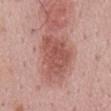<case>
<lighting>white-light</lighting>
<site>mid back</site>
<automated_metrics>
  <cielab_L>53</cielab_L>
  <cielab_a>25</cielab_a>
  <cielab_b>26</cielab_b>
  <vs_skin_darker_L>12.0</vs_skin_darker_L>
  <vs_skin_contrast_norm>8.0</vs_skin_contrast_norm>
  <nevus_likeness_0_100>75</nevus_likeness_0_100>
  <lesion_detection_confidence_0_100>100</lesion_detection_confidence_0_100>
</automated_metrics>
<patient>
  <sex>male</sex>
  <age_approx>45</age_approx>
</patient>
<image>
  <source>total-body photography crop</source>
  <field_of_view_mm>15</field_of_view_mm>
</image>
</case>